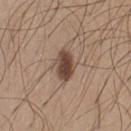The lesion was tiled from a total-body skin photograph and was not biopsied. Located on the chest. The tile uses white-light illumination. The recorded lesion diameter is about 4 mm. A 15 mm close-up extracted from a 3D total-body photography capture. A male subject, approximately 25 years of age.